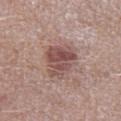This lesion was catalogued during total-body skin photography and was not selected for biopsy. Measured at roughly 5.5 mm in maximum diameter. Captured under white-light illumination. Located on the left lower leg. Cropped from a whole-body photographic skin survey; the tile spans about 15 mm. A male subject about 50 years old.